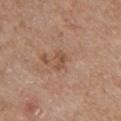Acquisition and patient details: Imaged with white-light lighting. The patient is a female aged around 85 or older. Located on the left upper arm. Longest diameter approximately 2.5 mm. Automated image analysis of the tile measured an average lesion color of about L≈51 a*≈20 b*≈31 (CIELAB) and a normalized lesion–skin contrast near 6. A 15 mm close-up tile from a total-body photography series done for melanoma screening.